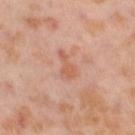Q: Was a biopsy performed?
A: imaged on a skin check; not biopsied
Q: Lesion size?
A: ~3.5 mm (longest diameter)
Q: What kind of image is this?
A: total-body-photography crop, ~15 mm field of view
Q: Lesion location?
A: the leg
Q: Automated lesion metrics?
A: a lesion color around L≈60 a*≈24 b*≈32 in CIELAB, a lesion–skin lightness drop of about 8, and a normalized border contrast of about 5.5; border irregularity of about 5.5 on a 0–10 scale, internal color variation of about 1 on a 0–10 scale, and radial color variation of about 0.5; a classifier nevus-likeness of about 0/100 and lesion-presence confidence of about 100/100
Q: Patient demographics?
A: female, aged approximately 55